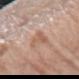<record>
<site>arm</site>
<patient>
  <sex>female</sex>
  <age_approx>70</age_approx>
</patient>
<image>
  <source>total-body photography crop</source>
  <field_of_view_mm>15</field_of_view_mm>
</image>
</record>